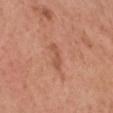Assessment: The lesion was photographed on a routine skin check and not biopsied; there is no pathology result. Image and clinical context: A male subject in their 60s. Cropped from a whole-body photographic skin survey; the tile spans about 15 mm. From the head or neck.